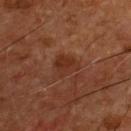Notes:
• biopsy status — no biopsy performed (imaged during a skin exam)
• patient — male, aged around 55
• automated lesion analysis — a lesion area of about 5.5 mm² and an eccentricity of roughly 0.55; a nevus-likeness score of about 5/100 and lesion-presence confidence of about 100/100
• imaging modality — total-body-photography crop, ~15 mm field of view
• site — the chest